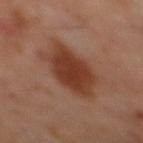This lesion was catalogued during total-body skin photography and was not selected for biopsy. A 15 mm close-up extracted from a 3D total-body photography capture. This is a cross-polarized tile. A male subject, aged approximately 60. Approximately 7.5 mm at its widest. Located on the mid back.